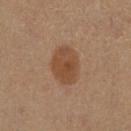follow-up=total-body-photography surveillance lesion; no biopsy | diameter=about 4.5 mm | subject=female, approximately 45 years of age | image=~15 mm tile from a whole-body skin photo | illumination=cross-polarized | location=the leg.